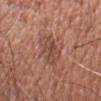The lesion's longest dimension is about 4 mm.
Captured under white-light illumination.
A region of skin cropped from a whole-body photographic capture, roughly 15 mm wide.
A male subject, in their mid- to late 60s.
The lesion is on the head or neck.